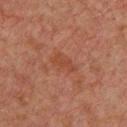* workup · no biopsy performed (imaged during a skin exam)
* location · the chest
* acquisition · 15 mm crop, total-body photography
* patient · male, aged 58–62